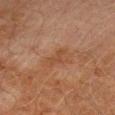The lesion was tiled from a total-body skin photograph and was not biopsied. A 15 mm close-up tile from a total-body photography series done for melanoma screening. On the arm. Measured at roughly 2.5 mm in maximum diameter. The tile uses cross-polarized illumination. A male subject aged approximately 70.